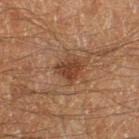{"biopsy_status": "not biopsied; imaged during a skin examination", "lighting": "cross-polarized", "image": {"source": "total-body photography crop", "field_of_view_mm": 15}, "site": "left thigh", "patient": {"sex": "male", "age_approx": 60}, "lesion_size": {"long_diameter_mm_approx": 3.0}}